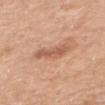Recorded during total-body skin imaging; not selected for excision or biopsy.
On the back.
A female subject, roughly 55 years of age.
This image is a 15 mm lesion crop taken from a total-body photograph.